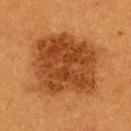| field | value |
|---|---|
| workup | total-body-photography surveillance lesion; no biopsy |
| imaging modality | total-body-photography crop, ~15 mm field of view |
| image-analysis metrics | an area of roughly 48 mm², an outline eccentricity of about 0.45 (0 = round, 1 = elongated), and a symmetry-axis asymmetry near 0.2; border irregularity of about 2 on a 0–10 scale, a color-variation rating of about 4.5/10, and a peripheral color-asymmetry measure near 2; lesion-presence confidence of about 100/100 |
| lighting | cross-polarized illumination |
| site | the upper back |
| patient | female, aged approximately 55 |